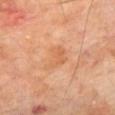body site: the front of the torso
automated metrics: a border-irregularity rating of about 4.5/10, a color-variation rating of about 2/10, and peripheral color asymmetry of about 1; a detector confidence of about 100 out of 100 that the crop contains a lesion
image source: total-body-photography crop, ~15 mm field of view
lesion size: ~3.5 mm (longest diameter)
patient: male, about 70 years old